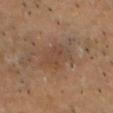This lesion was catalogued during total-body skin photography and was not selected for biopsy. The lesion is located on the head or neck. The tile uses cross-polarized illumination. A close-up tile cropped from a whole-body skin photograph, about 15 mm across. The subject is a male approximately 45 years of age.